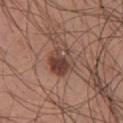The lesion was tiled from a total-body skin photograph and was not biopsied.
Imaged with white-light lighting.
Automated tile analysis of the lesion measured an area of roughly 9.5 mm², a shape eccentricity near 0.5, and a symmetry-axis asymmetry near 0.15. It also reported an automated nevus-likeness rating near 70 out of 100 and a detector confidence of about 100 out of 100 that the crop contains a lesion.
A male patient aged 28–32.
On the front of the torso.
The recorded lesion diameter is about 3.5 mm.
A close-up tile cropped from a whole-body skin photograph, about 15 mm across.